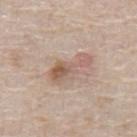biopsy status=imaged on a skin check; not biopsied | anatomic site=the abdomen | subject=male, aged 63–67 | imaging modality=~15 mm crop, total-body skin-cancer survey.